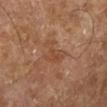Notes:
* workup: total-body-photography surveillance lesion; no biopsy
* image source: total-body-photography crop, ~15 mm field of view
* patient: in their mid- to late 60s
* lesion size: ~3.5 mm (longest diameter)
* location: the left lower leg
* lighting: cross-polarized